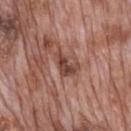{
  "biopsy_status": "not biopsied; imaged during a skin examination",
  "patient": {
    "sex": "male",
    "age_approx": 70
  },
  "site": "mid back",
  "image": {
    "source": "total-body photography crop",
    "field_of_view_mm": 15
  }
}